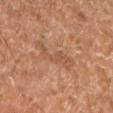* imaging modality — ~15 mm crop, total-body skin-cancer survey
* tile lighting — cross-polarized
* patient — in their mid-60s
* lesion diameter — ~5.5 mm (longest diameter)
* anatomic site — the left lower leg